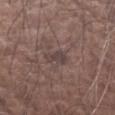workup = imaged on a skin check; not biopsied | subject = male, aged around 75 | lesion size = about 3.5 mm | image = total-body-photography crop, ~15 mm field of view | body site = the arm | tile lighting = white-light.